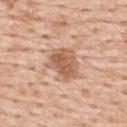follow-up = no biopsy performed (imaged during a skin exam)